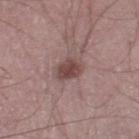  biopsy_status: not biopsied; imaged during a skin examination
  image:
    source: total-body photography crop
    field_of_view_mm: 15
  patient:
    sex: male
    age_approx: 60
  site: left thigh
  lesion_size:
    long_diameter_mm_approx: 3.0